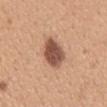workup = catalogued during a skin exam; not biopsied | imaging modality = ~15 mm crop, total-body skin-cancer survey | body site = the back | patient = female, roughly 30 years of age.